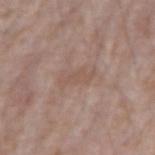Findings:
– biopsy status · imaged on a skin check; not biopsied
– subject · male, approximately 75 years of age
– body site · the right forearm
– automated metrics · a footprint of about 5 mm², a shape eccentricity near 0.9, and a shape-asymmetry score of about 0.35 (0 = symmetric); a border-irregularity rating of about 4.5/10, a within-lesion color-variation index near 1/10, and peripheral color asymmetry of about 0.5; a nevus-likeness score of about 0/100 and lesion-presence confidence of about 100/100
– lesion size · about 4 mm
– imaging modality · 15 mm crop, total-body photography